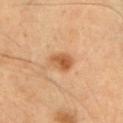Clinical impression: The lesion was photographed on a routine skin check and not biopsied; there is no pathology result.